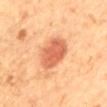| field | value |
|---|---|
| follow-up | catalogued during a skin exam; not biopsied |
| patient | female, aged approximately 60 |
| imaging modality | ~15 mm tile from a whole-body skin photo |
| location | the mid back |